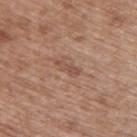Imaged during a routine full-body skin examination; the lesion was not biopsied and no histopathology is available. On the upper back. This image is a 15 mm lesion crop taken from a total-body photograph. A male patient roughly 50 years of age.